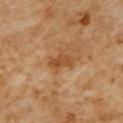Case summary:
- notes — no biopsy performed (imaged during a skin exam)
- subject — male, aged 58 to 62
- lighting — cross-polarized
- body site — the front of the torso
- imaging modality — 15 mm crop, total-body photography
- diameter — ~3 mm (longest diameter)